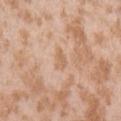workup=total-body-photography surveillance lesion; no biopsy
image source=~15 mm crop, total-body skin-cancer survey
site=the left upper arm
patient=female, aged approximately 25
lesion diameter=≈2.5 mm